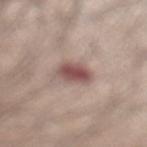Captured during whole-body skin photography for melanoma surveillance; the lesion was not biopsied.
Cropped from a whole-body photographic skin survey; the tile spans about 15 mm.
A male patient aged 33–37.
The lesion's longest dimension is about 3.5 mm.
The lesion is located on the left lower leg.
Captured under white-light illumination.